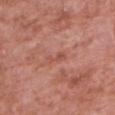notes: imaged on a skin check; not biopsied
subject: male, roughly 60 years of age
illumination: white-light illumination
image: ~15 mm crop, total-body skin-cancer survey
anatomic site: the left upper arm
lesion size: ~2.5 mm (longest diameter)